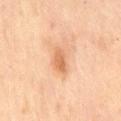The lesion was photographed on a routine skin check and not biopsied; there is no pathology result. Imaged with cross-polarized lighting. A female subject, aged 58–62. The lesion is located on the mid back. A close-up tile cropped from a whole-body skin photograph, about 15 mm across. Automated tile analysis of the lesion measured an average lesion color of about L≈59 a*≈22 b*≈35 (CIELAB). The software also gave a border-irregularity rating of about 2.5/10, internal color variation of about 3 on a 0–10 scale, and peripheral color asymmetry of about 1. It also reported an automated nevus-likeness rating near 35 out of 100 and a lesion-detection confidence of about 100/100. The recorded lesion diameter is about 3.5 mm.